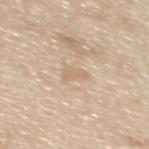The tile uses white-light illumination.
A female patient, aged 53 to 57.
The lesion-visualizer software estimated an average lesion color of about L≈66 a*≈15 b*≈32 (CIELAB), a lesion–skin lightness drop of about 7, and a lesion-to-skin contrast of about 5 (normalized; higher = more distinct). The software also gave a border-irregularity rating of about 4/10, a color-variation rating of about 0/10, and peripheral color asymmetry of about 0. And it measured a lesion-detection confidence of about 100/100.
This image is a 15 mm lesion crop taken from a total-body photograph.
Measured at roughly 2.5 mm in maximum diameter.
From the mid back.